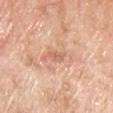Imaged during a routine full-body skin examination; the lesion was not biopsied and no histopathology is available.
Cropped from a whole-body photographic skin survey; the tile spans about 15 mm.
The lesion's longest dimension is about 2.5 mm.
Automated image analysis of the tile measured lesion-presence confidence of about 100/100.
A male patient, aged 63–67.
The lesion is on the chest.